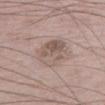Q: What kind of image is this?
A: total-body-photography crop, ~15 mm field of view
Q: Where on the body is the lesion?
A: the leg
Q: What did automated image analysis measure?
A: an eccentricity of roughly 0.85 and a symmetry-axis asymmetry near 0.25; a mean CIELAB color near L≈55 a*≈14 b*≈22, about 9 CIELAB-L* units darker than the surrounding skin, and a normalized border contrast of about 6.5; a border-irregularity rating of about 3/10, internal color variation of about 7 on a 0–10 scale, and a peripheral color-asymmetry measure near 3
Q: Patient demographics?
A: male, aged 73–77